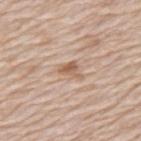Q: Was this lesion biopsied?
A: no biopsy performed (imaged during a skin exam)
Q: What is the lesion's diameter?
A: ~3 mm (longest diameter)
Q: What is the imaging modality?
A: ~15 mm crop, total-body skin-cancer survey
Q: Patient demographics?
A: male, in their 80s
Q: What is the anatomic site?
A: the mid back
Q: Illumination type?
A: white-light illumination